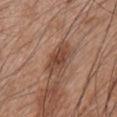Recorded during total-body skin imaging; not selected for excision or biopsy. A 15 mm close-up tile from a total-body photography series done for melanoma screening. The tile uses white-light illumination. The lesion is on the chest. A male patient roughly 45 years of age. The recorded lesion diameter is about 4 mm.